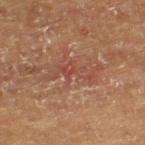Notes:
• workup: no biopsy performed (imaged during a skin exam)
• subject: male, aged approximately 65
• image source: 15 mm crop, total-body photography
• lesion size: ≈6.5 mm
• site: the left thigh
• illumination: cross-polarized